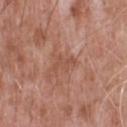Impression: Part of a total-body skin-imaging series; this lesion was reviewed on a skin check and was not flagged for biopsy. Context: Measured at roughly 3.5 mm in maximum diameter. The lesion is located on the upper back. Imaged with white-light lighting. A male patient, aged 48 to 52. A roughly 15 mm field-of-view crop from a total-body skin photograph. The lesion-visualizer software estimated an area of roughly 3.5 mm² and a shape eccentricity near 0.9. And it measured about 6 CIELAB-L* units darker than the surrounding skin and a normalized border contrast of about 5. The software also gave a border-irregularity rating of about 6/10, internal color variation of about 0.5 on a 0–10 scale, and radial color variation of about 0.5.